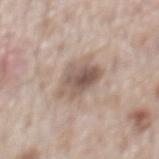Imaged during a routine full-body skin examination; the lesion was not biopsied and no histopathology is available. Imaged with white-light lighting. The patient is a male about 70 years old. A roughly 15 mm field-of-view crop from a total-body skin photograph. On the lower back. The lesion-visualizer software estimated a border-irregularity index near 3/10, internal color variation of about 7.5 on a 0–10 scale, and a peripheral color-asymmetry measure near 3. Measured at roughly 4 mm in maximum diameter.